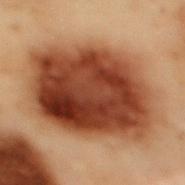biopsy status: total-body-photography surveillance lesion; no biopsy | body site: the mid back | diameter: about 10.5 mm | illumination: cross-polarized illumination | patient: male, aged approximately 55 | image: ~15 mm crop, total-body skin-cancer survey | automated lesion analysis: a mean CIELAB color near L≈35 a*≈22 b*≈29, a lesion–skin lightness drop of about 17, and a lesion-to-skin contrast of about 14 (normalized; higher = more distinct); border irregularity of about 2 on a 0–10 scale, a color-variation rating of about 8.5/10, and radial color variation of about 3.